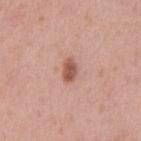Impression:
Captured during whole-body skin photography for melanoma surveillance; the lesion was not biopsied.
Image and clinical context:
The lesion is on the abdomen. This image is a 15 mm lesion crop taken from a total-body photograph. A male subject about 55 years old.